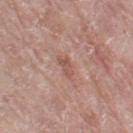Impression:
The lesion was photographed on a routine skin check and not biopsied; there is no pathology result.
Clinical summary:
A lesion tile, about 15 mm wide, cut from a 3D total-body photograph. Captured under white-light illumination. The lesion-visualizer software estimated a symmetry-axis asymmetry near 0.4. And it measured a normalized border contrast of about 6. It also reported a nevus-likeness score of about 0/100 and a lesion-detection confidence of about 100/100. The recorded lesion diameter is about 2.5 mm. The patient is a female aged around 60. From the left thigh.